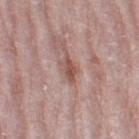The lesion was tiled from a total-body skin photograph and was not biopsied. The patient is a female aged around 70. On the leg. Measured at roughly 3 mm in maximum diameter. An algorithmic analysis of the crop reported an area of roughly 3 mm² and an outline eccentricity of about 0.9 (0 = round, 1 = elongated). The software also gave a border-irregularity index near 3.5/10 and a peripheral color-asymmetry measure near 0.5. The software also gave lesion-presence confidence of about 95/100. The tile uses white-light illumination. A 15 mm close-up tile from a total-body photography series done for melanoma screening.